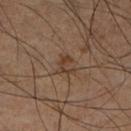Part of a total-body skin-imaging series; this lesion was reviewed on a skin check and was not flagged for biopsy.
The tile uses cross-polarized illumination.
The lesion is on the right lower leg.
The recorded lesion diameter is about 2.5 mm.
The total-body-photography lesion software estimated a lesion color around L≈37 a*≈15 b*≈26 in CIELAB, roughly 7 lightness units darker than nearby skin, and a normalized border contrast of about 6.5. And it measured a border-irregularity index near 6/10, a within-lesion color-variation index near 0.5/10, and a peripheral color-asymmetry measure near 0.5.
A male subject, in their 60s.
Cropped from a whole-body photographic skin survey; the tile spans about 15 mm.